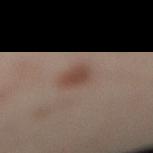- workup — no biopsy performed (imaged during a skin exam)
- automated lesion analysis — internal color variation of about 1.5 on a 0–10 scale and radial color variation of about 0.5; a nevus-likeness score of about 95/100 and a detector confidence of about 100 out of 100 that the crop contains a lesion
- subject — female, aged 38–42
- image source — 15 mm crop, total-body photography
- location — the leg
- tile lighting — cross-polarized
- diameter — ~2.5 mm (longest diameter)